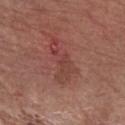Findings:
* follow-up · no biopsy performed (imaged during a skin exam)
* automated metrics · border irregularity of about 7 on a 0–10 scale, internal color variation of about 2 on a 0–10 scale, and a peripheral color-asymmetry measure near 0.5
* size · ~6 mm (longest diameter)
* image · 15 mm crop, total-body photography
* patient · male, aged approximately 75
* body site · the right forearm